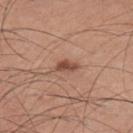Q: Was a biopsy performed?
A: catalogued during a skin exam; not biopsied
Q: Patient demographics?
A: male, about 35 years old
Q: How was this image acquired?
A: ~15 mm tile from a whole-body skin photo
Q: What is the anatomic site?
A: the left upper arm
Q: Automated lesion metrics?
A: a lesion area of about 3 mm², an eccentricity of roughly 0.85, and two-axis asymmetry of about 0.25; a lesion color around L≈48 a*≈23 b*≈28 in CIELAB, about 12 CIELAB-L* units darker than the surrounding skin, and a normalized border contrast of about 8.5